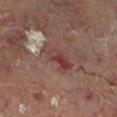follow-up: total-body-photography surveillance lesion; no biopsy
diameter: ≈4.5 mm
illumination: cross-polarized
location: the left lower leg
image source: ~15 mm tile from a whole-body skin photo